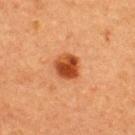{"biopsy_status": "not biopsied; imaged during a skin examination", "patient": {"sex": "male", "age_approx": 40}, "image": {"source": "total-body photography crop", "field_of_view_mm": 15}, "site": "upper back"}